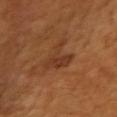Imaged during a routine full-body skin examination; the lesion was not biopsied and no histopathology is available.
From the right upper arm.
The tile uses cross-polarized illumination.
A female subject aged 53 to 57.
The lesion's longest dimension is about 4.5 mm.
A close-up tile cropped from a whole-body skin photograph, about 15 mm across.
The total-body-photography lesion software estimated a footprint of about 8.5 mm² and a shape-asymmetry score of about 0.7 (0 = symmetric). It also reported an average lesion color of about L≈38 a*≈24 b*≈34 (CIELAB) and a lesion–skin lightness drop of about 7.